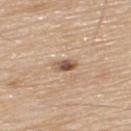<record>
<biopsy_status>not biopsied; imaged during a skin examination</biopsy_status>
<automated_metrics>
  <area_mm2_approx>3.5</area_mm2_approx>
  <eccentricity>0.8</eccentricity>
  <shape_asymmetry>0.25</shape_asymmetry>
  <border_irregularity_0_10>2.5</border_irregularity_0_10>
  <color_variation_0_10>6.5</color_variation_0_10>
</automated_metrics>
<site>upper back</site>
<lighting>white-light</lighting>
<patient>
  <sex>male</sex>
  <age_approx>80</age_approx>
</patient>
<lesion_size>
  <long_diameter_mm_approx>2.5</long_diameter_mm_approx>
</lesion_size>
<image>
  <source>total-body photography crop</source>
  <field_of_view_mm>15</field_of_view_mm>
</image>
</record>